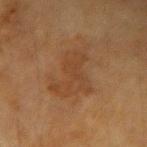<case>
<biopsy_status>not biopsied; imaged during a skin examination</biopsy_status>
<lesion_size>
  <long_diameter_mm_approx>6.0</long_diameter_mm_approx>
</lesion_size>
<patient>
  <sex>female</sex>
  <age_approx>55</age_approx>
</patient>
<site>left forearm</site>
<image>
  <source>total-body photography crop</source>
  <field_of_view_mm>15</field_of_view_mm>
</image>
<lighting>cross-polarized</lighting>
</case>